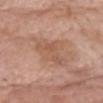follow-up=total-body-photography surveillance lesion; no biopsy.